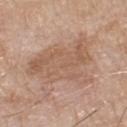Recorded during total-body skin imaging; not selected for excision or biopsy. Approximately 8 mm at its widest. On the chest. A male subject aged 78–82. A 15 mm close-up extracted from a 3D total-body photography capture.